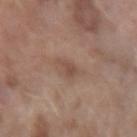| field | value |
|---|---|
| biopsy status | no biopsy performed (imaged during a skin exam) |
| image | ~15 mm tile from a whole-body skin photo |
| patient | female, aged approximately 60 |
| site | the left forearm |
| image-analysis metrics | a border-irregularity rating of about 3/10, a color-variation rating of about 1.5/10, and radial color variation of about 0.5 |